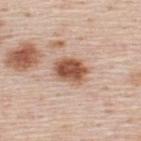Findings:
• notes — total-body-photography surveillance lesion; no biopsy
• image source — ~15 mm crop, total-body skin-cancer survey
• lighting — white-light illumination
• automated lesion analysis — a shape-asymmetry score of about 0.15 (0 = symmetric)
• location — the upper back
• size — ≈4 mm
• patient — male, approximately 45 years of age